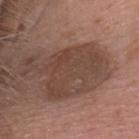Assessment: The lesion was photographed on a routine skin check and not biopsied; there is no pathology result. Clinical summary: Cropped from a whole-body photographic skin survey; the tile spans about 15 mm. The lesion is located on the head or neck. The recorded lesion diameter is about 7 mm. The lesion-visualizer software estimated border irregularity of about 7 on a 0–10 scale, internal color variation of about 3 on a 0–10 scale, and peripheral color asymmetry of about 1. A male subject aged approximately 55.